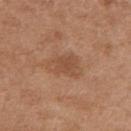– workup · imaged on a skin check; not biopsied
– image source · ~15 mm tile from a whole-body skin photo
– anatomic site · the mid back
– lighting · white-light illumination
– image-analysis metrics · an area of roughly 8.5 mm² and two-axis asymmetry of about 0.3
– lesion diameter · ≈4.5 mm
– subject · female, aged around 40